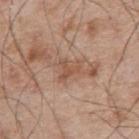Findings:
* biopsy status — imaged on a skin check; not biopsied
* image — ~15 mm tile from a whole-body skin photo
* automated metrics — a classifier nevus-likeness of about 0/100 and lesion-presence confidence of about 100/100
* subject — male, aged approximately 65
* diameter — about 3 mm
* anatomic site — the right upper arm
* tile lighting — white-light